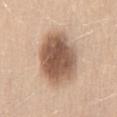Assessment:
No biopsy was performed on this lesion — it was imaged during a full skin examination and was not determined to be concerning.
Clinical summary:
An algorithmic analysis of the crop reported a lesion color around L≈56 a*≈18 b*≈29 in CIELAB, about 18 CIELAB-L* units darker than the surrounding skin, and a lesion-to-skin contrast of about 11 (normalized; higher = more distinct). Cropped from a whole-body photographic skin survey; the tile spans about 15 mm. Located on the lower back. A female subject roughly 55 years of age.